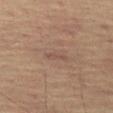workup = catalogued during a skin exam; not biopsied
patient = male, in their mid-60s
image = total-body-photography crop, ~15 mm field of view
automated metrics = a normalized border contrast of about 4.5; border irregularity of about 3.5 on a 0–10 scale and a peripheral color-asymmetry measure near 0
size = ~3 mm (longest diameter)
site = the left thigh
illumination = cross-polarized illumination